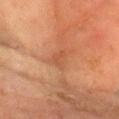On the head or neck.
The patient is a male roughly 65 years of age.
Cropped from a whole-body photographic skin survey; the tile spans about 15 mm.
The tile uses cross-polarized illumination.
The total-body-photography lesion software estimated a lesion area of about 3.5 mm², a shape eccentricity near 0.9, and a symmetry-axis asymmetry near 0.4. The analysis additionally found an automated nevus-likeness rating near 0 out of 100 and a lesion-detection confidence of about 95/100.
About 3 mm across.